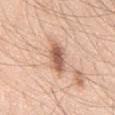Q: Was this lesion biopsied?
A: catalogued during a skin exam; not biopsied
Q: What is the anatomic site?
A: the chest
Q: What is the imaging modality?
A: total-body-photography crop, ~15 mm field of view
Q: What did automated image analysis measure?
A: border irregularity of about 3 on a 0–10 scale and a color-variation rating of about 3.5/10; a classifier nevus-likeness of about 95/100
Q: What is the lesion's diameter?
A: about 4.5 mm
Q: Who is the patient?
A: male, aged approximately 45